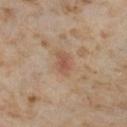Impression: This lesion was catalogued during total-body skin photography and was not selected for biopsy. Context: The recorded lesion diameter is about 3 mm. Imaged with cross-polarized lighting. A region of skin cropped from a whole-body photographic capture, roughly 15 mm wide. A female patient roughly 55 years of age. Located on the left lower leg.